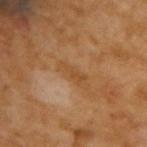Clinical impression:
Recorded during total-body skin imaging; not selected for excision or biopsy.
Image and clinical context:
A male patient aged around 65. This image is a 15 mm lesion crop taken from a total-body photograph.